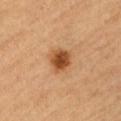<record>
  <biopsy_status>not biopsied; imaged during a skin examination</biopsy_status>
  <lesion_size>
    <long_diameter_mm_approx>3.0</long_diameter_mm_approx>
  </lesion_size>
  <site>front of the torso</site>
  <lighting>cross-polarized</lighting>
  <patient>
    <sex>male</sex>
    <age_approx>85+</age_approx>
  </patient>
  <image>
    <source>total-body photography crop</source>
    <field_of_view_mm>15</field_of_view_mm>
  </image>
</record>